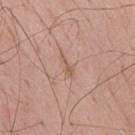Assessment: Captured during whole-body skin photography for melanoma surveillance; the lesion was not biopsied. Acquisition and patient details: Automated image analysis of the tile measured a lesion color around L≈58 a*≈20 b*≈29 in CIELAB and roughly 7 lightness units darker than nearby skin. It also reported border irregularity of about 4.5 on a 0–10 scale, internal color variation of about 0 on a 0–10 scale, and a peripheral color-asymmetry measure near 0. And it measured an automated nevus-likeness rating near 0 out of 100 and a lesion-detection confidence of about 70/100. A close-up tile cropped from a whole-body skin photograph, about 15 mm across. A male subject, aged 63 to 67. The lesion is located on the upper back. This is a white-light tile. Measured at roughly 3 mm in maximum diameter.